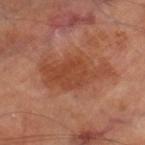notes: total-body-photography surveillance lesion; no biopsy | lesion diameter: ≈7.5 mm | acquisition: total-body-photography crop, ~15 mm field of view | body site: the left thigh | patient: male, roughly 70 years of age | illumination: cross-polarized illumination.